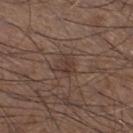No biopsy was performed on this lesion — it was imaged during a full skin examination and was not determined to be concerning. A male patient in their 60s. A 15 mm crop from a total-body photograph taken for skin-cancer surveillance. The total-body-photography lesion software estimated a classifier nevus-likeness of about 5/100 and lesion-presence confidence of about 90/100. On the left lower leg. Longest diameter approximately 2.5 mm.